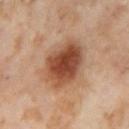Located on the right thigh.
Captured under cross-polarized illumination.
Longest diameter approximately 6 mm.
A close-up tile cropped from a whole-body skin photograph, about 15 mm across.
A female patient in their mid-50s.